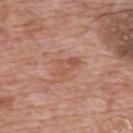biopsy status=imaged on a skin check; not biopsied | body site=the upper back | subject=male, aged 68 to 72 | diameter=about 3.5 mm | image=~15 mm crop, total-body skin-cancer survey | automated lesion analysis=roughly 7 lightness units darker than nearby skin and a normalized border contrast of about 5.5; a nevus-likeness score of about 0/100 and a lesion-detection confidence of about 100/100.